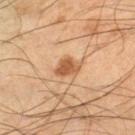biopsy_status: not biopsied; imaged during a skin examination
image:
  source: total-body photography crop
  field_of_view_mm: 15
lesion_size:
  long_diameter_mm_approx: 3.5
lighting: cross-polarized
patient:
  sex: male
  age_approx: 50
site: leg